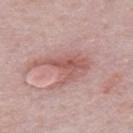notes — catalogued during a skin exam; not biopsied | patient — male, aged 48 to 52 | imaging modality — ~15 mm tile from a whole-body skin photo | anatomic site — the upper back | size — about 6.5 mm.